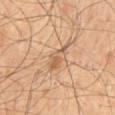{
  "biopsy_status": "not biopsied; imaged during a skin examination",
  "patient": {
    "sex": "male",
    "age_approx": 55
  },
  "site": "mid back",
  "image": {
    "source": "total-body photography crop",
    "field_of_view_mm": 15
  }
}